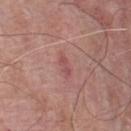Notes:
– workup: total-body-photography surveillance lesion; no biopsy
– illumination: white-light
– subject: male, approximately 80 years of age
– diameter: about 3 mm
– anatomic site: the chest
– image: ~15 mm tile from a whole-body skin photo